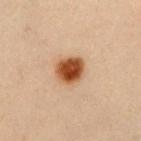The lesion was photographed on a routine skin check and not biopsied; there is no pathology result.
Measured at roughly 3.5 mm in maximum diameter.
A 15 mm crop from a total-body photograph taken for skin-cancer surveillance.
A female patient, in their 40s.
The lesion is located on the chest.
This is a cross-polarized tile.